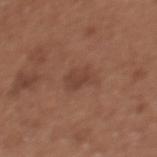Cropped from a whole-body photographic skin survey; the tile spans about 15 mm. A female patient in their mid-30s. The lesion's longest dimension is about 3.5 mm. This is a white-light tile. From the chest.